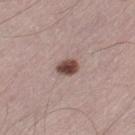Captured during whole-body skin photography for melanoma surveillance; the lesion was not biopsied. The tile uses white-light illumination. The subject is a male aged 43–47. The lesion is on the left thigh. A region of skin cropped from a whole-body photographic capture, roughly 15 mm wide. The lesion's longest dimension is about 3 mm. Automated image analysis of the tile measured a border-irregularity rating of about 1.5/10, a within-lesion color-variation index near 3.5/10, and peripheral color asymmetry of about 1.